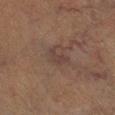Impression: The lesion was photographed on a routine skin check and not biopsied; there is no pathology result. Background: The subject is a male about 85 years old or older. The lesion-visualizer software estimated an automated nevus-likeness rating near 0 out of 100 and a lesion-detection confidence of about 95/100. Located on the leg. This image is a 15 mm lesion crop taken from a total-body photograph.